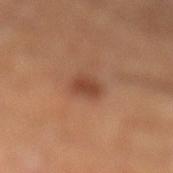Case summary:
* notes · imaged on a skin check; not biopsied
* patient · male, aged around 50
* imaging modality · ~15 mm crop, total-body skin-cancer survey
* lighting · cross-polarized
* automated lesion analysis · a lesion area of about 4.5 mm² and an eccentricity of roughly 0.55; an average lesion color of about L≈41 a*≈23 b*≈30 (CIELAB), roughly 9 lightness units darker than nearby skin, and a normalized border contrast of about 7.5; a border-irregularity index near 2/10, a color-variation rating of about 2/10, and a peripheral color-asymmetry measure near 0.5
* diameter · about 2.5 mm
* anatomic site · the left lower leg